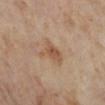patient = female, roughly 55 years of age | site = the leg | tile lighting = cross-polarized illumination | image = 15 mm crop, total-body photography | TBP lesion metrics = a detector confidence of about 100 out of 100 that the crop contains a lesion | lesion size = about 3.5 mm.